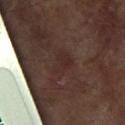Acquisition and patient details:
The patient is a female aged 78–82. From the left arm. A lesion tile, about 15 mm wide, cut from a 3D total-body photograph.